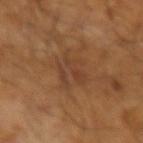Case summary:
• notes — no biopsy performed (imaged during a skin exam)
• diameter — ~4.5 mm (longest diameter)
• tile lighting — cross-polarized illumination
• TBP lesion metrics — a lesion area of about 9 mm², an outline eccentricity of about 0.75 (0 = round, 1 = elongated), and a shape-asymmetry score of about 0.4 (0 = symmetric); a mean CIELAB color near L≈39 a*≈20 b*≈30, about 6 CIELAB-L* units darker than the surrounding skin, and a normalized lesion–skin contrast near 5.5; an automated nevus-likeness rating near 0 out of 100 and lesion-presence confidence of about 85/100
• subject — male, in their mid-60s
• acquisition — total-body-photography crop, ~15 mm field of view